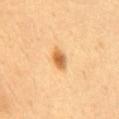{"lesion_size": {"long_diameter_mm_approx": 3.0}, "lighting": "cross-polarized", "image": {"source": "total-body photography crop", "field_of_view_mm": 15}, "patient": {"sex": "female", "age_approx": 40}, "automated_metrics": {"area_mm2_approx": 4.0, "eccentricity": 0.8, "shape_asymmetry": 0.15, "cielab_L": 65, "cielab_a": 23, "cielab_b": 46, "vs_skin_darker_L": 14.0, "vs_skin_contrast_norm": 9.0, "border_irregularity_0_10": 1.5, "color_variation_0_10": 4.0, "peripheral_color_asymmetry": 1.0}, "site": "back"}